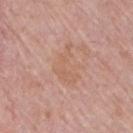Findings:
– notes: imaged on a skin check; not biopsied
– imaging modality: ~15 mm crop, total-body skin-cancer survey
– body site: the right upper arm
– diameter: ~5 mm (longest diameter)
– illumination: white-light
– patient: male, approximately 75 years of age